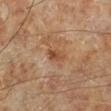Impression: Part of a total-body skin-imaging series; this lesion was reviewed on a skin check and was not flagged for biopsy. Image and clinical context: A lesion tile, about 15 mm wide, cut from a 3D total-body photograph. Imaged with cross-polarized lighting. Approximately 2.5 mm at its widest. The lesion is located on the left lower leg. The patient is a male aged 68 to 72.